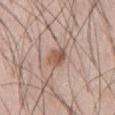notes=total-body-photography surveillance lesion; no biopsy | acquisition=15 mm crop, total-body photography | anatomic site=the abdomen | automated lesion analysis=a shape eccentricity near 0.65 and two-axis asymmetry of about 0.3; border irregularity of about 3 on a 0–10 scale, internal color variation of about 3 on a 0–10 scale, and peripheral color asymmetry of about 1.5; an automated nevus-likeness rating near 95 out of 100 and lesion-presence confidence of about 100/100 | diameter=~3 mm (longest diameter) | illumination=white-light illumination | patient=male, roughly 60 years of age.